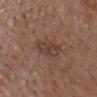<lesion>
<biopsy_status>not biopsied; imaged during a skin examination</biopsy_status>
<patient>
  <sex>male</sex>
  <age_approx>55</age_approx>
</patient>
<image>
  <source>total-body photography crop</source>
  <field_of_view_mm>15</field_of_view_mm>
</image>
<lighting>white-light</lighting>
<lesion_size>
  <long_diameter_mm_approx>3.5</long_diameter_mm_approx>
</lesion_size>
<site>head or neck</site>
</lesion>